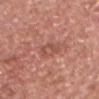biopsy_status: not biopsied; imaged during a skin examination
automated_metrics:
  color_variation_0_10: 4.0
  peripheral_color_asymmetry: 1.5
  nevus_likeness_0_100: 0
  lesion_detection_confidence_0_100: 100
lighting: white-light
patient:
  sex: male
  age_approx: 80
lesion_size:
  long_diameter_mm_approx: 3.0
image:
  source: total-body photography crop
  field_of_view_mm: 15
site: chest